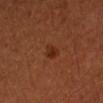workup — total-body-photography surveillance lesion; no biopsy
automated lesion analysis — lesion-presence confidence of about 100/100
subject — female, aged 48–52
image — total-body-photography crop, ~15 mm field of view
body site — the left forearm